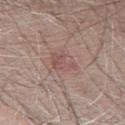<lesion>
  <biopsy_status>not biopsied; imaged during a skin examination</biopsy_status>
  <image>
    <source>total-body photography crop</source>
    <field_of_view_mm>15</field_of_view_mm>
  </image>
  <site>arm</site>
  <lesion_size>
    <long_diameter_mm_approx>3.0</long_diameter_mm_approx>
  </lesion_size>
  <patient>
    <sex>male</sex>
    <age_approx>65</age_approx>
  </patient>
  <lighting>white-light</lighting>
  <automated_metrics>
    <eccentricity>0.65</eccentricity>
    <shape_asymmetry>0.45</shape_asymmetry>
    <peripheral_color_asymmetry>1.0</peripheral_color_asymmetry>
    <nevus_likeness_0_100>5</nevus_likeness_0_100>
    <lesion_detection_confidence_0_100>100</lesion_detection_confidence_0_100>
  </automated_metrics>
</lesion>